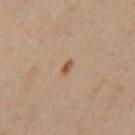Clinical impression:
The lesion was tiled from a total-body skin photograph and was not biopsied.
Acquisition and patient details:
Located on the right thigh. This image is a 15 mm lesion crop taken from a total-body photograph. Imaged with cross-polarized lighting. A female subject roughly 40 years of age. Measured at roughly 2 mm in maximum diameter.